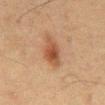{"site": "abdomen", "lighting": "cross-polarized", "patient": {"sex": "male", "age_approx": 60}, "image": {"source": "total-body photography crop", "field_of_view_mm": 15}, "lesion_size": {"long_diameter_mm_approx": 4.0}}